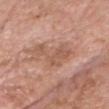Assessment:
This lesion was catalogued during total-body skin photography and was not selected for biopsy.
Image and clinical context:
The patient is a male approximately 75 years of age. The recorded lesion diameter is about 5.5 mm. Imaged with white-light lighting. Located on the head or neck. Cropped from a whole-body photographic skin survey; the tile spans about 15 mm.